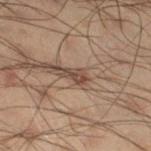biopsy status = catalogued during a skin exam; not biopsied | acquisition = ~15 mm tile from a whole-body skin photo | patient = male, about 50 years old | illumination = cross-polarized | body site = the leg.